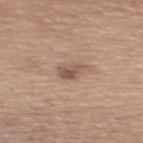The lesion was photographed on a routine skin check and not biopsied; there is no pathology result.
A female subject roughly 55 years of age.
The lesion is on the upper back.
This is a white-light tile.
A close-up tile cropped from a whole-body skin photograph, about 15 mm across.
The recorded lesion diameter is about 3 mm.